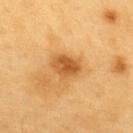notes: no biopsy performed (imaged during a skin exam) | lesion diameter: about 3.5 mm | patient: male, aged 58–62 | imaging modality: ~15 mm crop, total-body skin-cancer survey | site: the upper back | lighting: cross-polarized.